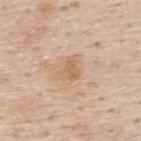Part of a total-body skin-imaging series; this lesion was reviewed on a skin check and was not flagged for biopsy. Cropped from a total-body skin-imaging series; the visible field is about 15 mm. A male subject approximately 45 years of age. Located on the upper back.